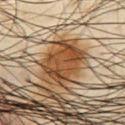Image and clinical context: A roughly 15 mm field-of-view crop from a total-body skin photograph. A male patient, approximately 65 years of age. Captured under cross-polarized illumination. Longest diameter approximately 7 mm. From the chest.